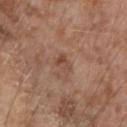Recorded during total-body skin imaging; not selected for excision or biopsy. Imaged with white-light lighting. A male patient, aged 73–77. On the left forearm. An algorithmic analysis of the crop reported a lesion color around L≈47 a*≈20 b*≈29 in CIELAB and about 8 CIELAB-L* units darker than the surrounding skin. And it measured a color-variation rating of about 2/10. And it measured a detector confidence of about 100 out of 100 that the crop contains a lesion. The recorded lesion diameter is about 2.5 mm. Cropped from a whole-body photographic skin survey; the tile spans about 15 mm.